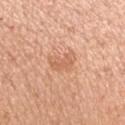follow-up: imaged on a skin check; not biopsied | location: the arm | diameter: ~3 mm (longest diameter) | subject: female, aged 38 to 42 | lighting: white-light illumination | image: ~15 mm tile from a whole-body skin photo.